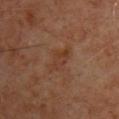<case>
  <biopsy_status>not biopsied; imaged during a skin examination</biopsy_status>
</case>